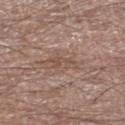Captured during whole-body skin photography for melanoma surveillance; the lesion was not biopsied.
The tile uses white-light illumination.
The lesion's longest dimension is about 3.5 mm.
On the left lower leg.
The patient is a male aged approximately 65.
Cropped from a whole-body photographic skin survey; the tile spans about 15 mm.